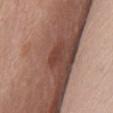anatomic site: the front of the torso
tile lighting: white-light
automated lesion analysis: a mean CIELAB color near L≈41 a*≈23 b*≈26, a lesion–skin lightness drop of about 8, and a lesion-to-skin contrast of about 6.5 (normalized; higher = more distinct); internal color variation of about 1 on a 0–10 scale and peripheral color asymmetry of about 0.5
subject: female, aged around 80
image source: total-body-photography crop, ~15 mm field of view
lesion diameter: about 3 mm